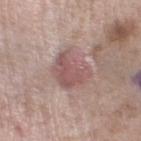A 15 mm close-up tile from a total-body photography series done for melanoma screening.
Imaged with white-light lighting.
The subject is a female approximately 55 years of age.
Automated image analysis of the tile measured a lesion area of about 13 mm² and a shape-asymmetry score of about 0.25 (0 = symmetric). The analysis additionally found a border-irregularity rating of about 3/10 and a within-lesion color-variation index near 4/10. It also reported a nevus-likeness score of about 25/100 and a lesion-detection confidence of about 95/100.
Located on the left lower leg.
Measured at roughly 4.5 mm in maximum diameter.
The biopsy diagnosis was a lichen planus-like keratosis — a benign skin lesion.